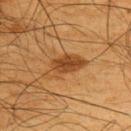Recorded during total-body skin imaging; not selected for excision or biopsy.
The lesion-visualizer software estimated a border-irregularity index near 3.5/10, a color-variation rating of about 3.5/10, and peripheral color asymmetry of about 1.5. It also reported a nevus-likeness score of about 90/100 and lesion-presence confidence of about 100/100.
A 15 mm close-up tile from a total-body photography series done for melanoma screening.
The lesion is located on the upper back.
A male subject roughly 65 years of age.
Measured at roughly 5 mm in maximum diameter.